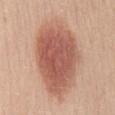Impression:
The lesion was tiled from a total-body skin photograph and was not biopsied.
Context:
A female subject, approximately 45 years of age. Located on the mid back. Captured under white-light illumination. A 15 mm crop from a total-body photograph taken for skin-cancer surveillance. The lesion's longest dimension is about 10.5 mm. The lesion-visualizer software estimated a border-irregularity index near 2/10.